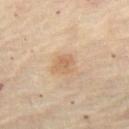Case summary:
* biopsy status · catalogued during a skin exam; not biopsied
* site · the left thigh
* subject · female, in their 60s
* acquisition · total-body-photography crop, ~15 mm field of view
* tile lighting · cross-polarized
* size · ≈3 mm